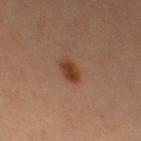Impression:
No biopsy was performed on this lesion — it was imaged during a full skin examination and was not determined to be concerning.
Context:
The tile uses cross-polarized illumination. The recorded lesion diameter is about 2.5 mm. A region of skin cropped from a whole-body photographic capture, roughly 15 mm wide. The subject is a female in their 50s. The lesion is located on the left thigh.